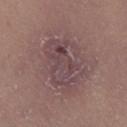<lesion>
<biopsy_status>not biopsied; imaged during a skin examination</biopsy_status>
<image>
  <source>total-body photography crop</source>
  <field_of_view_mm>15</field_of_view_mm>
</image>
<patient>
  <sex>female</sex>
  <age_approx>20</age_approx>
</patient>
<lighting>white-light</lighting>
<site>left lower leg</site>
<lesion_size>
  <long_diameter_mm_approx>8.5</long_diameter_mm_approx>
</lesion_size>
</lesion>